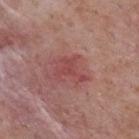This lesion was catalogued during total-body skin photography and was not selected for biopsy. Imaged with white-light lighting. A male subject, roughly 70 years of age. Located on the upper back. A lesion tile, about 15 mm wide, cut from a 3D total-body photograph. About 3.5 mm across.